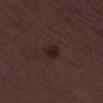workup: catalogued during a skin exam; not biopsied | tile lighting: white-light illumination | acquisition: 15 mm crop, total-body photography | diameter: ~2.5 mm (longest diameter) | automated metrics: a footprint of about 4 mm² and two-axis asymmetry of about 0.2; an average lesion color of about L≈20 a*≈16 b*≈15 (CIELAB), a lesion–skin lightness drop of about 6, and a lesion-to-skin contrast of about 8 (normalized; higher = more distinct); a border-irregularity index near 1.5/10 and a within-lesion color-variation index near 2/10 | patient: male, aged 48 to 52.